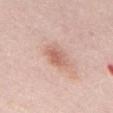| feature | finding |
|---|---|
| notes | no biopsy performed (imaged during a skin exam) |
| image source | ~15 mm crop, total-body skin-cancer survey |
| subject | male, aged 48 to 52 |
| size | ~3 mm (longest diameter) |
| site | the abdomen |
| illumination | white-light |